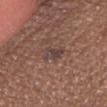{
  "biopsy_status": "not biopsied; imaged during a skin examination",
  "site": "head or neck",
  "lighting": "white-light",
  "lesion_size": {
    "long_diameter_mm_approx": 3.0
  },
  "patient": {
    "sex": "male",
    "age_approx": 65
  },
  "automated_metrics": {
    "area_mm2_approx": 5.0,
    "eccentricity": 0.75,
    "shape_asymmetry": 0.3,
    "color_variation_0_10": 4.0
  },
  "image": {
    "source": "total-body photography crop",
    "field_of_view_mm": 15
  }
}